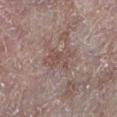The lesion is located on the leg.
A female subject, about 65 years old.
Cropped from a total-body skin-imaging series; the visible field is about 15 mm.